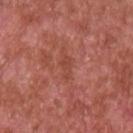Captured during whole-body skin photography for melanoma surveillance; the lesion was not biopsied.
On the chest.
Measured at roughly 3 mm in maximum diameter.
Automated image analysis of the tile measured a shape eccentricity near 0.9. The analysis additionally found a lesion color around L≈46 a*≈29 b*≈30 in CIELAB, roughly 6 lightness units darker than nearby skin, and a lesion-to-skin contrast of about 5 (normalized; higher = more distinct). And it measured a border-irregularity rating of about 4/10, a color-variation rating of about 1/10, and radial color variation of about 0.5.
A male subject, aged 63 to 67.
Cropped from a whole-body photographic skin survey; the tile spans about 15 mm.
This is a white-light tile.